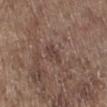Assessment: The lesion was tiled from a total-body skin photograph and was not biopsied. Clinical summary: This is a white-light tile. The recorded lesion diameter is about 2.5 mm. A male patient, aged approximately 70. A 15 mm crop from a total-body photograph taken for skin-cancer surveillance. The lesion is located on the right lower leg.